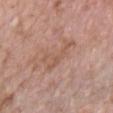Impression: Part of a total-body skin-imaging series; this lesion was reviewed on a skin check and was not flagged for biopsy. Context: The lesion is located on the chest. The tile uses white-light illumination. A close-up tile cropped from a whole-body skin photograph, about 15 mm across. The patient is a female aged 73–77. The lesion-visualizer software estimated a footprint of about 6 mm², an outline eccentricity of about 0.95 (0 = round, 1 = elongated), and a shape-asymmetry score of about 0.7 (0 = symmetric). The software also gave a border-irregularity rating of about 9/10 and a within-lesion color-variation index near 2.5/10. The analysis additionally found an automated nevus-likeness rating near 0 out of 100 and lesion-presence confidence of about 70/100.